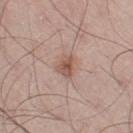The lesion was tiled from a total-body skin photograph and was not biopsied.
Located on the left thigh.
Measured at roughly 3 mm in maximum diameter.
The patient is a male approximately 55 years of age.
A 15 mm crop from a total-body photograph taken for skin-cancer surveillance.